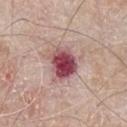Captured during whole-body skin photography for melanoma surveillance; the lesion was not biopsied. The lesion-visualizer software estimated a footprint of about 11 mm², a shape eccentricity near 0.55, and a shape-asymmetry score of about 0.2 (0 = symmetric). It also reported an average lesion color of about L≈48 a*≈29 b*≈17 (CIELAB) and a lesion–skin lightness drop of about 19. And it measured a border-irregularity index near 2/10, internal color variation of about 7.5 on a 0–10 scale, and radial color variation of about 2. The analysis additionally found an automated nevus-likeness rating near 0 out of 100 and a detector confidence of about 100 out of 100 that the crop contains a lesion. Located on the chest. A 15 mm crop from a total-body photograph taken for skin-cancer surveillance. A male patient aged approximately 65. The recorded lesion diameter is about 4 mm. The tile uses white-light illumination.